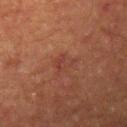Imaged during a routine full-body skin examination; the lesion was not biopsied and no histopathology is available. A roughly 15 mm field-of-view crop from a total-body skin photograph. Approximately 2.5 mm at its widest. From the left upper arm. A male subject aged approximately 55. Imaged with cross-polarized lighting. The total-body-photography lesion software estimated a shape-asymmetry score of about 0.55 (0 = symmetric). It also reported a lesion–skin lightness drop of about 5 and a normalized border contrast of about 5. It also reported a color-variation rating of about 2/10 and a peripheral color-asymmetry measure near 1.